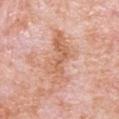biopsy_status: not biopsied; imaged during a skin examination
image:
  source: total-body photography crop
  field_of_view_mm: 15
automated_metrics:
  area_mm2_approx: 13.0
  eccentricity: 0.9
  shape_asymmetry: 0.5
  cielab_L: 63
  cielab_a: 24
  cielab_b: 33
  vs_skin_darker_L: 9.0
  vs_skin_contrast_norm: 6.5
  border_irregularity_0_10: 8.0
  color_variation_0_10: 4.0
  nevus_likeness_0_100: 0
  lesion_detection_confidence_0_100: 100
lesion_size:
  long_diameter_mm_approx: 6.5
patient:
  sex: male
  age_approx: 80
lighting: white-light
site: chest